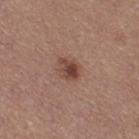Impression: This lesion was catalogued during total-body skin photography and was not selected for biopsy. Image and clinical context: The lesion is located on the right thigh. A close-up tile cropped from a whole-body skin photograph, about 15 mm across. A female patient, aged around 35.